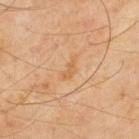Q: Was this lesion biopsied?
A: catalogued during a skin exam; not biopsied
Q: What are the patient's age and sex?
A: male, aged around 45
Q: Illumination type?
A: cross-polarized illumination
Q: How large is the lesion?
A: about 3 mm
Q: Lesion location?
A: the upper back
Q: How was this image acquired?
A: ~15 mm tile from a whole-body skin photo